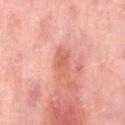follow-up — total-body-photography surveillance lesion; no biopsy
subject — female, aged 48 to 52
image source — ~15 mm crop, total-body skin-cancer survey
anatomic site — the abdomen